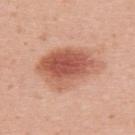Case summary:
- biopsy status — imaged on a skin check; not biopsied
- image-analysis metrics — an average lesion color of about L≈57 a*≈26 b*≈31 (CIELAB), about 14 CIELAB-L* units darker than the surrounding skin, and a lesion-to-skin contrast of about 9 (normalized; higher = more distinct); a border-irregularity rating of about 2.5/10 and radial color variation of about 2
- illumination — white-light illumination
- lesion size — ≈7.5 mm
- body site — the upper back
- patient — female, aged around 30
- image — total-body-photography crop, ~15 mm field of view